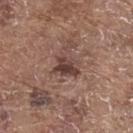Clinical impression:
This lesion was catalogued during total-body skin photography and was not selected for biopsy.
Acquisition and patient details:
About 3.5 mm across. A male patient, approximately 80 years of age. The lesion is on the back. A close-up tile cropped from a whole-body skin photograph, about 15 mm across. The lesion-visualizer software estimated a shape eccentricity near 0.65 and two-axis asymmetry of about 0.4. And it measured an average lesion color of about L≈40 a*≈19 b*≈22 (CIELAB) and a lesion–skin lightness drop of about 11. And it measured an automated nevus-likeness rating near 5 out of 100 and lesion-presence confidence of about 100/100.